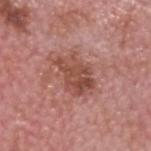Imaged during a routine full-body skin examination; the lesion was not biopsied and no histopathology is available. Cropped from a total-body skin-imaging series; the visible field is about 15 mm. Approximately 5 mm at its widest. A male patient aged 73–77. The total-body-photography lesion software estimated an area of roughly 12 mm² and a symmetry-axis asymmetry near 0.3. The analysis additionally found a lesion color around L≈49 a*≈25 b*≈27 in CIELAB, roughly 10 lightness units darker than nearby skin, and a normalized lesion–skin contrast near 7.5. The analysis additionally found an automated nevus-likeness rating near 5 out of 100 and a lesion-detection confidence of about 100/100. The lesion is located on the head or neck.